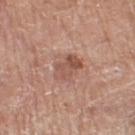{
  "biopsy_status": "not biopsied; imaged during a skin examination",
  "patient": {
    "sex": "female",
    "age_approx": 75
  },
  "image": {
    "source": "total-body photography crop",
    "field_of_view_mm": 15
  },
  "site": "left thigh",
  "lesion_size": {
    "long_diameter_mm_approx": 3.5
  },
  "lighting": "white-light"
}